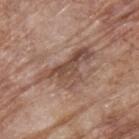Q: Is there a histopathology result?
A: no biopsy performed (imaged during a skin exam)
Q: What is the lesion's diameter?
A: ≈7 mm
Q: What kind of image is this?
A: 15 mm crop, total-body photography
Q: Lesion location?
A: the upper back
Q: What are the patient's age and sex?
A: male, roughly 70 years of age
Q: How was the tile lit?
A: white-light illumination